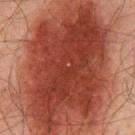Q: Was this lesion biopsied?
A: no biopsy performed (imaged during a skin exam)
Q: Where on the body is the lesion?
A: the chest
Q: How was this image acquired?
A: total-body-photography crop, ~15 mm field of view
Q: What are the patient's age and sex?
A: male, aged approximately 60